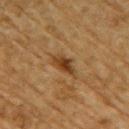lighting: cross-polarized
body site: the right upper arm
patient: male, in their mid- to late 80s or older
image: 15 mm crop, total-body photography
lesion size: ~3.5 mm (longest diameter)
TBP lesion metrics: an average lesion color of about L≈35 a*≈18 b*≈32 (CIELAB), about 10 CIELAB-L* units darker than the surrounding skin, and a lesion-to-skin contrast of about 9 (normalized; higher = more distinct); a color-variation rating of about 3.5/10